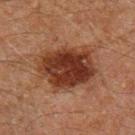Findings:
* notes — total-body-photography surveillance lesion; no biopsy
* lesion diameter — ~6.5 mm (longest diameter)
* illumination — cross-polarized
* site — the right thigh
* subject — male, approximately 60 years of age
* imaging modality — 15 mm crop, total-body photography